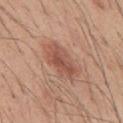A male subject, roughly 40 years of age. Imaged with white-light lighting. An algorithmic analysis of the crop reported a border-irregularity index near 3/10, internal color variation of about 4 on a 0–10 scale, and peripheral color asymmetry of about 1.5. On the mid back. About 5 mm across. A 15 mm close-up extracted from a 3D total-body photography capture.